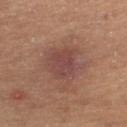The lesion was photographed on a routine skin check and not biopsied; there is no pathology result. A female subject aged 43 to 47. Longest diameter approximately 3.5 mm. Captured under cross-polarized illumination. The lesion is on the right lower leg. Cropped from a total-body skin-imaging series; the visible field is about 15 mm.